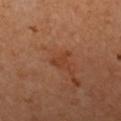Clinical impression: The lesion was tiled from a total-body skin photograph and was not biopsied. Context: From the left forearm. Automated image analysis of the tile measured a lesion–skin lightness drop of about 6 and a normalized border contrast of about 6. And it measured a nevus-likeness score of about 0/100. The lesion's longest dimension is about 2.5 mm. A region of skin cropped from a whole-body photographic capture, roughly 15 mm wide. Captured under cross-polarized illumination. A female patient, about 50 years old.